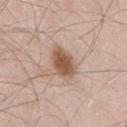Impression: Imaged during a routine full-body skin examination; the lesion was not biopsied and no histopathology is available. Image and clinical context: Imaged with white-light lighting. The lesion's longest dimension is about 4 mm. The subject is a male approximately 45 years of age. From the right thigh. Cropped from a total-body skin-imaging series; the visible field is about 15 mm. The total-body-photography lesion software estimated a footprint of about 8 mm², an eccentricity of roughly 0.65, and two-axis asymmetry of about 0.15.